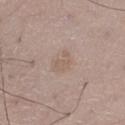No biopsy was performed on this lesion — it was imaged during a full skin examination and was not determined to be concerning. This is a white-light tile. Approximately 3 mm at its widest. Cropped from a total-body skin-imaging series; the visible field is about 15 mm. The patient is a male in their 50s. The lesion is located on the leg.